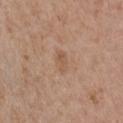follow-up=no biopsy performed (imaged during a skin exam)
subject=female, aged around 65
size=~2.5 mm (longest diameter)
imaging modality=total-body-photography crop, ~15 mm field of view
image-analysis metrics=a footprint of about 3.5 mm², an eccentricity of roughly 0.85, and two-axis asymmetry of about 0.2; a lesion color around L≈55 a*≈19 b*≈31 in CIELAB and a normalized border contrast of about 5; lesion-presence confidence of about 100/100
site=the chest